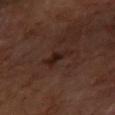<tbp_lesion>
  <biopsy_status>not biopsied; imaged during a skin examination</biopsy_status>
  <lesion_size>
    <long_diameter_mm_approx>3.0</long_diameter_mm_approx>
  </lesion_size>
  <image>
    <source>total-body photography crop</source>
    <field_of_view_mm>15</field_of_view_mm>
  </image>
  <site>left forearm</site>
  <lighting>cross-polarized</lighting>
  <patient>
    <sex>male</sex>
    <age_approx>65</age_approx>
  </patient>
  <automated_metrics>
    <border_irregularity_0_10>5.0</border_irregularity_0_10>
    <color_variation_0_10>2.0</color_variation_0_10>
    <peripheral_color_asymmetry>0.5</peripheral_color_asymmetry>
  </automated_metrics>
</tbp_lesion>